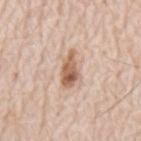Assessment: Captured during whole-body skin photography for melanoma surveillance; the lesion was not biopsied. Background: A lesion tile, about 15 mm wide, cut from a 3D total-body photograph. The subject is a male aged approximately 80. The lesion is on the mid back.